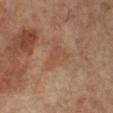The lesion was tiled from a total-body skin photograph and was not biopsied. Located on the left leg. The tile uses cross-polarized illumination. The lesion's longest dimension is about 3.5 mm. The total-body-photography lesion software estimated a within-lesion color-variation index near 3/10 and radial color variation of about 1. A female patient in their mid- to late 60s. A 15 mm close-up tile from a total-body photography series done for melanoma screening.